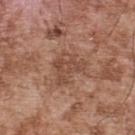Impression: No biopsy was performed on this lesion — it was imaged during a full skin examination and was not determined to be concerning. Image and clinical context: A male subject, about 55 years old. Located on the upper back. Automated image analysis of the tile measured two-axis asymmetry of about 0.45. The analysis additionally found a mean CIELAB color near L≈47 a*≈21 b*≈28, about 8 CIELAB-L* units darker than the surrounding skin, and a normalized border contrast of about 5.5. The analysis additionally found a border-irregularity rating of about 5/10 and internal color variation of about 3 on a 0–10 scale. The software also gave a classifier nevus-likeness of about 0/100. A 15 mm crop from a total-body photograph taken for skin-cancer surveillance. The lesion's longest dimension is about 4.5 mm.